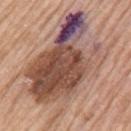Recorded during total-body skin imaging; not selected for excision or biopsy. This image is a 15 mm lesion crop taken from a total-body photograph. Automated image analysis of the tile measured an area of roughly 70 mm² and a symmetry-axis asymmetry near 0.3. The analysis additionally found a mean CIELAB color near L≈51 a*≈20 b*≈25, roughly 14 lightness units darker than nearby skin, and a normalized border contrast of about 10. It also reported internal color variation of about 10 on a 0–10 scale and radial color variation of about 6. And it measured an automated nevus-likeness rating near 5 out of 100 and a detector confidence of about 100 out of 100 that the crop contains a lesion. Located on the left upper arm. The patient is a male aged 58 to 62. Imaged with white-light lighting.